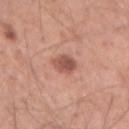workup=no biopsy performed (imaged during a skin exam)
diameter=≈3 mm
lighting=white-light illumination
subject=male, aged around 35
location=the left forearm
imaging modality=~15 mm tile from a whole-body skin photo